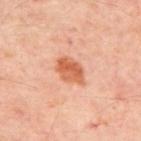notes=no biopsy performed (imaged during a skin exam) | diameter=≈4 mm | tile lighting=cross-polarized illumination | site=the upper back | image=total-body-photography crop, ~15 mm field of view | subject=about 55 years old.